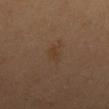Recorded during total-body skin imaging; not selected for excision or biopsy. From the mid back. A 15 mm crop from a total-body photograph taken for skin-cancer surveillance. Captured under cross-polarized illumination. The subject is a male roughly 50 years of age.